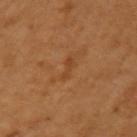Cropped from a whole-body photographic skin survey; the tile spans about 15 mm.
The total-body-photography lesion software estimated border irregularity of about 6 on a 0–10 scale and a within-lesion color-variation index near 0/10. The analysis additionally found a classifier nevus-likeness of about 0/100 and a detector confidence of about 100 out of 100 that the crop contains a lesion.
The patient is a female in their mid- to late 50s.
The tile uses cross-polarized illumination.
The lesion is located on the left upper arm.